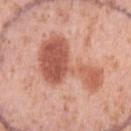site = the arm | image source = ~15 mm tile from a whole-body skin photo | illumination = white-light | patient = female, aged 38–42 | size = ≈8.5 mm | histopathologic diagnosis = a compound melanocytic nevus — a benign skin lesion.